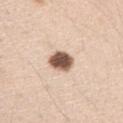The lesion was photographed on a routine skin check and not biopsied; there is no pathology result.
A female patient, aged around 40.
The lesion is located on the arm.
Cropped from a whole-body photographic skin survey; the tile spans about 15 mm.